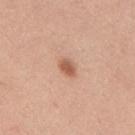Acquisition and patient details:
Imaged with white-light lighting. A male subject, aged 28 to 32. A 15 mm crop from a total-body photograph taken for skin-cancer surveillance. On the mid back. Longest diameter approximately 2.5 mm.